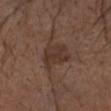{"image": {"source": "total-body photography crop", "field_of_view_mm": 15}, "site": "head or neck", "patient": {"sex": "male", "age_approx": 75}, "lighting": "white-light", "lesion_size": {"long_diameter_mm_approx": 3.5}}